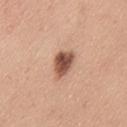<lesion>
  <biopsy_status>not biopsied; imaged during a skin examination</biopsy_status>
  <lesion_size>
    <long_diameter_mm_approx>4.0</long_diameter_mm_approx>
  </lesion_size>
  <automated_metrics>
    <area_mm2_approx>6.5</area_mm2_approx>
    <cielab_L>53</cielab_L>
    <cielab_a>22</cielab_a>
    <cielab_b>29</cielab_b>
    <vs_skin_darker_L>17.0</vs_skin_darker_L>
    <vs_skin_contrast_norm>11.0</vs_skin_contrast_norm>
    <nevus_likeness_0_100>100</nevus_likeness_0_100>
    <lesion_detection_confidence_0_100>100</lesion_detection_confidence_0_100>
  </automated_metrics>
  <lighting>white-light</lighting>
  <patient>
    <sex>female</sex>
    <age_approx>45</age_approx>
  </patient>
  <site>left thigh</site>
  <image>
    <source>total-body photography crop</source>
    <field_of_view_mm>15</field_of_view_mm>
  </image>
</lesion>